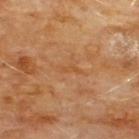Clinical impression:
This lesion was catalogued during total-body skin photography and was not selected for biopsy.
Acquisition and patient details:
The subject is a male aged 58–62. A close-up tile cropped from a whole-body skin photograph, about 15 mm across. Captured under cross-polarized illumination. The lesion's longest dimension is about 2.5 mm. Located on the chest.